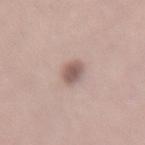workup = no biopsy performed (imaged during a skin exam)
anatomic site = the back
imaging modality = ~15 mm crop, total-body skin-cancer survey
patient = female, aged 48–52